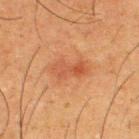illumination = cross-polarized illumination
lesion size = ~4 mm (longest diameter)
image source = total-body-photography crop, ~15 mm field of view
anatomic site = the upper back
TBP lesion metrics = a mean CIELAB color near L≈42 a*≈23 b*≈32, a lesion–skin lightness drop of about 7, and a normalized lesion–skin contrast near 6; border irregularity of about 3 on a 0–10 scale and radial color variation of about 1.5
patient = male, aged 33 to 37